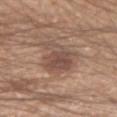Clinical impression: Part of a total-body skin-imaging series; this lesion was reviewed on a skin check and was not flagged for biopsy. Acquisition and patient details: A male subject, aged 18–22. The lesion-visualizer software estimated a border-irregularity index near 4.5/10 and a within-lesion color-variation index near 4.5/10. About 5.5 mm across. A lesion tile, about 15 mm wide, cut from a 3D total-body photograph. On the arm.